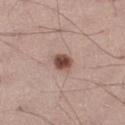Impression: Part of a total-body skin-imaging series; this lesion was reviewed on a skin check and was not flagged for biopsy. Context: A 15 mm crop from a total-body photograph taken for skin-cancer surveillance. The subject is a male in their mid-40s. Approximately 2.5 mm at its widest. Located on the left thigh.